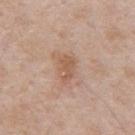<case>
  <biopsy_status>not biopsied; imaged during a skin examination</biopsy_status>
  <patient>
    <sex>male</sex>
    <age_approx>50</age_approx>
  </patient>
  <lesion_size>
    <long_diameter_mm_approx>3.0</long_diameter_mm_approx>
  </lesion_size>
  <lighting>white-light</lighting>
  <site>mid back</site>
  <image>
    <source>total-body photography crop</source>
    <field_of_view_mm>15</field_of_view_mm>
  </image>
</case>